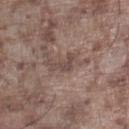Recorded during total-body skin imaging; not selected for excision or biopsy.
The subject is a male aged 73 to 77.
A 15 mm close-up extracted from a 3D total-body photography capture.
The lesion is on the left lower leg.